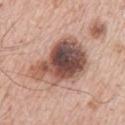biopsy_status: not biopsied; imaged during a skin examination
patient:
  sex: male
  age_approx: 65
image:
  source: total-body photography crop
  field_of_view_mm: 15
site: arm
lighting: white-light